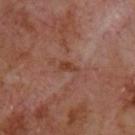Impression: Captured during whole-body skin photography for melanoma surveillance; the lesion was not biopsied. Acquisition and patient details: Captured under cross-polarized illumination. A roughly 15 mm field-of-view crop from a total-body skin photograph. The lesion is on the upper back. The recorded lesion diameter is about 2.5 mm. A male patient, aged 68–72.